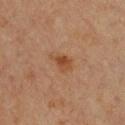Case summary:
* follow-up: catalogued during a skin exam; not biopsied
* image: ~15 mm tile from a whole-body skin photo
* anatomic site: the front of the torso
* subject: female, aged 43–47
* size: ~3 mm (longest diameter)
* lighting: cross-polarized
* image-analysis metrics: an average lesion color of about L≈40 a*≈20 b*≈31 (CIELAB) and a lesion–skin lightness drop of about 7; border irregularity of about 3.5 on a 0–10 scale, internal color variation of about 3 on a 0–10 scale, and a peripheral color-asymmetry measure near 1; an automated nevus-likeness rating near 80 out of 100 and a detector confidence of about 100 out of 100 that the crop contains a lesion